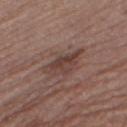No biopsy was performed on this lesion — it was imaged during a full skin examination and was not determined to be concerning. Longest diameter approximately 4.5 mm. This is a white-light tile. Located on the left thigh. The total-body-photography lesion software estimated a lesion area of about 8 mm², an outline eccentricity of about 0.8 (0 = round, 1 = elongated), and a shape-asymmetry score of about 0.4 (0 = symmetric). The software also gave a lesion color around L≈40 a*≈18 b*≈22 in CIELAB, a lesion–skin lightness drop of about 9, and a normalized lesion–skin contrast near 7.5. It also reported border irregularity of about 4.5 on a 0–10 scale, a color-variation rating of about 5/10, and a peripheral color-asymmetry measure near 2. The software also gave a nevus-likeness score of about 10/100 and a detector confidence of about 100 out of 100 that the crop contains a lesion. A region of skin cropped from a whole-body photographic capture, roughly 15 mm wide. A male subject aged around 65.